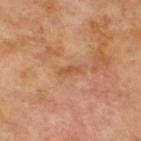Assessment: The lesion was tiled from a total-body skin photograph and was not biopsied. Image and clinical context: Located on the upper back. Measured at roughly 2.5 mm in maximum diameter. A close-up tile cropped from a whole-body skin photograph, about 15 mm across. The lesion-visualizer software estimated an automated nevus-likeness rating near 0 out of 100 and lesion-presence confidence of about 100/100. The tile uses cross-polarized illumination. A male patient, aged 68 to 72.